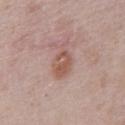Image and clinical context:
Measured at roughly 4.5 mm in maximum diameter. The tile uses white-light illumination. From the chest. A lesion tile, about 15 mm wide, cut from a 3D total-body photograph. A male patient aged approximately 40. The total-body-photography lesion software estimated a border-irregularity rating of about 3.5/10.